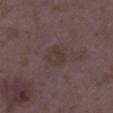The lesion was photographed on a routine skin check and not biopsied; there is no pathology result.